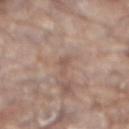Imaged during a routine full-body skin examination; the lesion was not biopsied and no histopathology is available.
The lesion is on the mid back.
Captured under white-light illumination.
A male subject, aged around 80.
Cropped from a total-body skin-imaging series; the visible field is about 15 mm.
Measured at roughly 3 mm in maximum diameter.